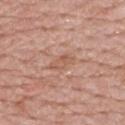Notes:
• follow-up · imaged on a skin check; not biopsied
• site · the mid back
• size · ~3.5 mm (longest diameter)
• tile lighting · white-light
• image-analysis metrics · a footprint of about 5 mm² and an eccentricity of roughly 0.85; an average lesion color of about L≈57 a*≈22 b*≈30 (CIELAB), roughly 7 lightness units darker than nearby skin, and a normalized lesion–skin contrast near 5; a border-irregularity rating of about 3/10, a within-lesion color-variation index near 3.5/10, and a peripheral color-asymmetry measure near 1.5
• patient · male, about 50 years old
• acquisition · ~15 mm tile from a whole-body skin photo